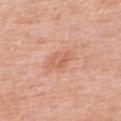{
  "biopsy_status": "not biopsied; imaged during a skin examination",
  "lesion_size": {
    "long_diameter_mm_approx": 3.0
  },
  "image": {
    "source": "total-body photography crop",
    "field_of_view_mm": 15
  },
  "lighting": "white-light",
  "site": "upper back",
  "patient": {
    "sex": "female",
    "age_approx": 50
  },
  "automated_metrics": {
    "area_mm2_approx": 4.0,
    "shape_asymmetry": 0.4
  }
}